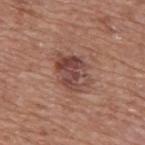The lesion was tiled from a total-body skin photograph and was not biopsied. A male subject, approximately 75 years of age. From the back. A region of skin cropped from a whole-body photographic capture, roughly 15 mm wide. This is a white-light tile. The total-body-photography lesion software estimated a lesion area of about 13 mm², a shape eccentricity near 0.7, and two-axis asymmetry of about 0.25. The software also gave a mean CIELAB color near L≈45 a*≈22 b*≈24, a lesion–skin lightness drop of about 10, and a lesion-to-skin contrast of about 8 (normalized; higher = more distinct).